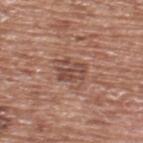No biopsy was performed on this lesion — it was imaged during a full skin examination and was not determined to be concerning. This image is a 15 mm lesion crop taken from a total-body photograph. From the back. A male patient aged 73–77.